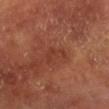| field | value |
|---|---|
| notes | total-body-photography surveillance lesion; no biopsy |
| size | about 3 mm |
| body site | the left lower leg |
| patient | male, aged approximately 55 |
| automated lesion analysis | a footprint of about 3.5 mm², an outline eccentricity of about 0.85 (0 = round, 1 = elongated), and a shape-asymmetry score of about 0.65 (0 = symmetric); internal color variation of about 0 on a 0–10 scale; a nevus-likeness score of about 0/100 and a detector confidence of about 100 out of 100 that the crop contains a lesion |
| image source | ~15 mm crop, total-body skin-cancer survey |